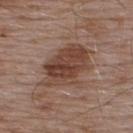  biopsy_status: not biopsied; imaged during a skin examination